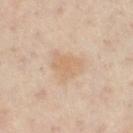Findings:
* biopsy status · catalogued during a skin exam; not biopsied
* image source · total-body-photography crop, ~15 mm field of view
* patient · female, roughly 40 years of age
* anatomic site · the left leg
* size · ≈4 mm
* tile lighting · cross-polarized